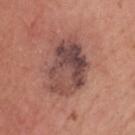Impression:
Recorded during total-body skin imaging; not selected for excision or biopsy.
Acquisition and patient details:
The tile uses white-light illumination. Located on the head or neck. A 15 mm crop from a total-body photograph taken for skin-cancer surveillance. The recorded lesion diameter is about 6.5 mm. A male subject, about 60 years old.